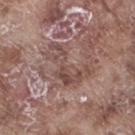Imaged during a routine full-body skin examination; the lesion was not biopsied and no histopathology is available.
Longest diameter approximately 6 mm.
From the right thigh.
A lesion tile, about 15 mm wide, cut from a 3D total-body photograph.
This is a white-light tile.
Automated tile analysis of the lesion measured a lesion color around L≈48 a*≈18 b*≈22 in CIELAB, roughly 8 lightness units darker than nearby skin, and a normalized lesion–skin contrast near 6. The analysis additionally found a border-irregularity rating of about 10/10, internal color variation of about 5 on a 0–10 scale, and radial color variation of about 2. It also reported a classifier nevus-likeness of about 0/100.
A male patient in their mid- to late 70s.